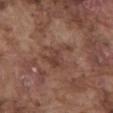Case summary:
- workup: imaged on a skin check; not biopsied
- tile lighting: white-light
- automated metrics: border irregularity of about 7.5 on a 0–10 scale and radial color variation of about 1
- acquisition: 15 mm crop, total-body photography
- anatomic site: the front of the torso
- subject: male, about 75 years old